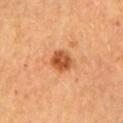Q: Was a biopsy performed?
A: total-body-photography surveillance lesion; no biopsy